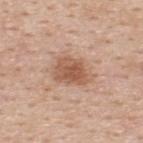The lesion was photographed on a routine skin check and not biopsied; there is no pathology result. A 15 mm close-up extracted from a 3D total-body photography capture. From the upper back. The total-body-photography lesion software estimated a lesion area of about 11 mm², an outline eccentricity of about 0.75 (0 = round, 1 = elongated), and two-axis asymmetry of about 0.25. And it measured border irregularity of about 3 on a 0–10 scale, a color-variation rating of about 3.5/10, and radial color variation of about 1. The software also gave an automated nevus-likeness rating near 90 out of 100 and a lesion-detection confidence of about 100/100. A male subject, aged 48–52. The recorded lesion diameter is about 4.5 mm.